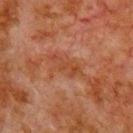Captured during whole-body skin photography for melanoma surveillance; the lesion was not biopsied. A 15 mm close-up tile from a total-body photography series done for melanoma screening. The subject is a male aged 78 to 82. This is a cross-polarized tile. From the chest.